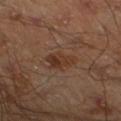Clinical impression:
This lesion was catalogued during total-body skin photography and was not selected for biopsy.
Acquisition and patient details:
Located on the left lower leg. The recorded lesion diameter is about 3.5 mm. A male patient, approximately 45 years of age. A roughly 15 mm field-of-view crop from a total-body skin photograph.